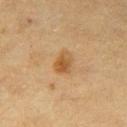No biopsy was performed on this lesion — it was imaged during a full skin examination and was not determined to be concerning. The subject is a female aged 53 to 57. This image is a 15 mm lesion crop taken from a total-body photograph. The tile uses cross-polarized illumination. Located on the right thigh. About 3 mm across.